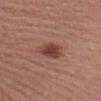Q: Was this lesion biopsied?
A: no biopsy performed (imaged during a skin exam)
Q: How was this image acquired?
A: ~15 mm tile from a whole-body skin photo
Q: What are the patient's age and sex?
A: female, roughly 55 years of age
Q: Where on the body is the lesion?
A: the left upper arm
Q: What lighting was used for the tile?
A: white-light illumination
Q: How large is the lesion?
A: ~3 mm (longest diameter)